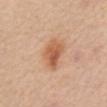Clinical impression: The lesion was photographed on a routine skin check and not biopsied; there is no pathology result. Background: The lesion is located on the abdomen. The patient is a female aged approximately 70. A 15 mm close-up extracted from a 3D total-body photography capture. Longest diameter approximately 4 mm. This is a white-light tile.